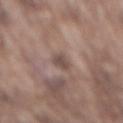{"image": {"source": "total-body photography crop", "field_of_view_mm": 15}, "site": "mid back", "lesion_size": {"long_diameter_mm_approx": 2.5}, "automated_metrics": {"cielab_L": 48, "cielab_a": 16, "cielab_b": 21, "border_irregularity_0_10": 2.5, "color_variation_0_10": 1.0, "peripheral_color_asymmetry": 0.5}, "patient": {"sex": "male", "age_approx": 75}, "lighting": "white-light"}